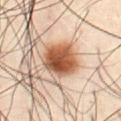Clinical impression:
No biopsy was performed on this lesion — it was imaged during a full skin examination and was not determined to be concerning.
Context:
The lesion is on the abdomen. Imaged with cross-polarized lighting. A 15 mm close-up tile from a total-body photography series done for melanoma screening. A male patient, aged approximately 50.